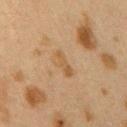The lesion was photographed on a routine skin check and not biopsied; there is no pathology result. A roughly 15 mm field-of-view crop from a total-body skin photograph. A female patient aged 38 to 42. The recorded lesion diameter is about 3.5 mm. The total-body-photography lesion software estimated a footprint of about 5 mm², a shape eccentricity near 0.85, and a symmetry-axis asymmetry near 0.25. And it measured an automated nevus-likeness rating near 0 out of 100 and a lesion-detection confidence of about 100/100. The lesion is located on the right upper arm. This is a cross-polarized tile.